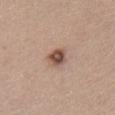biopsy_status: not biopsied; imaged during a skin examination
image:
  source: total-body photography crop
  field_of_view_mm: 15
site: chest
lesion_size:
  long_diameter_mm_approx: 2.5
automated_metrics:
  area_mm2_approx: 4.5
  cielab_L: 50
  cielab_a: 19
  cielab_b: 26
  vs_skin_contrast_norm: 10.0
  nevus_likeness_0_100: 90
  lesion_detection_confidence_0_100: 100
patient:
  sex: female
  age_approx: 40
lighting: white-light